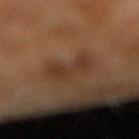location = the right lower leg; image = ~15 mm crop, total-body skin-cancer survey; subject = male, aged 58–62.